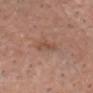image: ~15 mm tile from a whole-body skin photo | body site: the chest | automated metrics: an area of roughly 3 mm², a shape eccentricity near 0.85, and a symmetry-axis asymmetry near 0.4; a lesion color around L≈49 a*≈21 b*≈28 in CIELAB and a normalized lesion–skin contrast near 6; border irregularity of about 3.5 on a 0–10 scale, a within-lesion color-variation index near 1.5/10, and peripheral color asymmetry of about 0.5; a classifier nevus-likeness of about 0/100 and lesion-presence confidence of about 100/100 | patient: male, about 75 years old | size: about 2.5 mm.